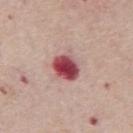acquisition — ~15 mm crop, total-body skin-cancer survey | anatomic site — the mid back | lesion diameter — about 4 mm | patient — male, roughly 75 years of age | lighting — white-light.